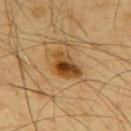workup=imaged on a skin check; not biopsied | anatomic site=the upper back | automated metrics=a lesion area of about 9 mm², a shape eccentricity near 0.75, and a symmetry-axis asymmetry near 0.35; a mean CIELAB color near L≈48 a*≈20 b*≈41, roughly 13 lightness units darker than nearby skin, and a normalized lesion–skin contrast near 9.5; a border-irregularity index near 3.5/10 and internal color variation of about 10 on a 0–10 scale; an automated nevus-likeness rating near 85 out of 100 and a detector confidence of about 100 out of 100 that the crop contains a lesion | diameter=~4.5 mm (longest diameter) | tile lighting=cross-polarized illumination | imaging modality=~15 mm tile from a whole-body skin photo | subject=male, aged around 65.